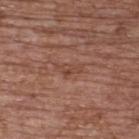notes = no biopsy performed (imaged during a skin exam)
site = the back
lighting = white-light illumination
diameter = ~3 mm (longest diameter)
acquisition = ~15 mm tile from a whole-body skin photo
patient = female, aged 63 to 67